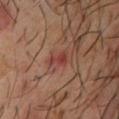workup: no biopsy performed (imaged during a skin exam)
body site: the chest
lighting: cross-polarized illumination
subject: male, approximately 60 years of age
image-analysis metrics: a footprint of about 3 mm², a shape eccentricity near 0.85, and two-axis asymmetry of about 0.45; a lesion-to-skin contrast of about 6.5 (normalized; higher = more distinct); a border-irregularity index near 4.5/10, a color-variation rating of about 1/10, and peripheral color asymmetry of about 0.5; a classifier nevus-likeness of about 0/100 and a lesion-detection confidence of about 100/100
size: ≈3 mm
image: ~15 mm crop, total-body skin-cancer survey